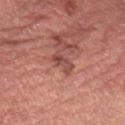{"biopsy_status": "not biopsied; imaged during a skin examination", "patient": {"sex": "male", "age_approx": 70}, "lesion_size": {"long_diameter_mm_approx": 3.0}, "image": {"source": "total-body photography crop", "field_of_view_mm": 15}, "site": "lower back"}